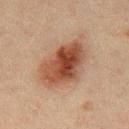Part of a total-body skin-imaging series; this lesion was reviewed on a skin check and was not flagged for biopsy. A male subject aged 48 to 52. On the mid back. About 6.5 mm across. This image is a 15 mm lesion crop taken from a total-body photograph. The tile uses cross-polarized illumination.